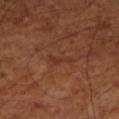patient:
  age_approx: 65
image:
  source: total-body photography crop
  field_of_view_mm: 15
site: leg